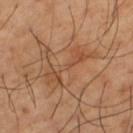Part of a total-body skin-imaging series; this lesion was reviewed on a skin check and was not flagged for biopsy. The lesion is on the left thigh. The subject is a male aged around 65. A 15 mm close-up extracted from a 3D total-body photography capture.